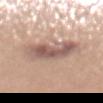Captured during whole-body skin photography for melanoma surveillance; the lesion was not biopsied. Located on the mid back. An algorithmic analysis of the crop reported a lesion area of about 19 mm², a shape eccentricity near 0.8, and a symmetry-axis asymmetry near 0.25. The software also gave an average lesion color of about L≈58 a*≈18 b*≈24 (CIELAB), about 11 CIELAB-L* units darker than the surrounding skin, and a lesion-to-skin contrast of about 8 (normalized; higher = more distinct). And it measured a border-irregularity index near 3.5/10, a color-variation rating of about 6.5/10, and a peripheral color-asymmetry measure near 2.5. And it measured an automated nevus-likeness rating near 5 out of 100 and a lesion-detection confidence of about 100/100. About 7 mm across. This image is a 15 mm lesion crop taken from a total-body photograph. A female subject, aged around 55.